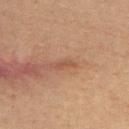follow-up=catalogued during a skin exam; not biopsied
tile lighting=cross-polarized
image source=15 mm crop, total-body photography
lesion size=about 2.5 mm
subject=female, aged around 45
anatomic site=the upper back